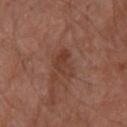Image and clinical context: The total-body-photography lesion software estimated a mean CIELAB color near L≈37 a*≈21 b*≈26, about 7 CIELAB-L* units darker than the surrounding skin, and a normalized border contrast of about 6.5. And it measured a classifier nevus-likeness of about 0/100 and a lesion-detection confidence of about 100/100. This image is a 15 mm lesion crop taken from a total-body photograph. The subject is a male roughly 70 years of age. From the left lower leg.